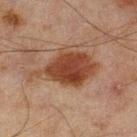- workup: imaged on a skin check; not biopsied